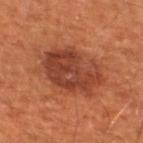follow-up = total-body-photography surveillance lesion; no biopsy
image-analysis metrics = a lesion area of about 26 mm², an eccentricity of roughly 0.7, and a symmetry-axis asymmetry near 0.15; a border-irregularity index near 2.5/10, a within-lesion color-variation index near 5.5/10, and radial color variation of about 2
lesion diameter = about 7 mm
illumination = cross-polarized illumination
location = the left thigh
patient = in their mid- to late 60s
acquisition = ~15 mm crop, total-body skin-cancer survey